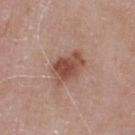Part of a total-body skin-imaging series; this lesion was reviewed on a skin check and was not flagged for biopsy.
The lesion-visualizer software estimated a border-irregularity rating of about 2.5/10 and radial color variation of about 1. The analysis additionally found a nevus-likeness score of about 90/100 and lesion-presence confidence of about 100/100.
Located on the chest.
The patient is a male aged approximately 75.
About 4 mm across.
A 15 mm crop from a total-body photograph taken for skin-cancer surveillance.
Captured under white-light illumination.